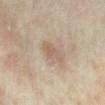Notes:
• notes: total-body-photography surveillance lesion; no biopsy
• imaging modality: 15 mm crop, total-body photography
• site: the arm
• tile lighting: cross-polarized
• patient: female, aged 33–37